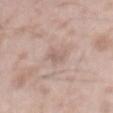Notes:
* workup · catalogued during a skin exam; not biopsied
* patient · male, about 50 years old
* image source · 15 mm crop, total-body photography
* location · the mid back